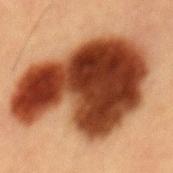This lesion was catalogued during total-body skin photography and was not selected for biopsy. This is a cross-polarized tile. Measured at roughly 12.5 mm in maximum diameter. A 15 mm crop from a total-body photograph taken for skin-cancer surveillance. A male patient aged approximately 55. The lesion is located on the abdomen.